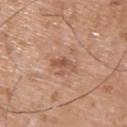Imaged during a routine full-body skin examination; the lesion was not biopsied and no histopathology is available.
A male patient, approximately 50 years of age.
A 15 mm crop from a total-body photograph taken for skin-cancer surveillance.
Captured under white-light illumination.
Located on the upper back.